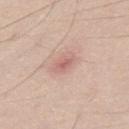image-analysis metrics = a nevus-likeness score of about 0/100; diameter = about 2.5 mm; subject = male, roughly 35 years of age; tile lighting = white-light illumination; acquisition = 15 mm crop, total-body photography; site = the mid back.